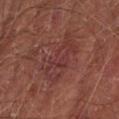Assessment: Recorded during total-body skin imaging; not selected for excision or biopsy. Clinical summary: A 15 mm close-up extracted from a 3D total-body photography capture. The lesion is located on the left forearm. Imaged with cross-polarized lighting. Automated tile analysis of the lesion measured a mean CIELAB color near L≈34 a*≈23 b*≈21, about 5 CIELAB-L* units darker than the surrounding skin, and a lesion-to-skin contrast of about 5.5 (normalized; higher = more distinct). It also reported a border-irregularity index near 5/10 and a peripheral color-asymmetry measure near 1.5. The analysis additionally found a classifier nevus-likeness of about 0/100 and a detector confidence of about 90 out of 100 that the crop contains a lesion. The subject is a male aged approximately 65. Measured at roughly 6 mm in maximum diameter.